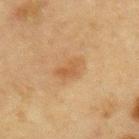<lesion>
<biopsy_status>not biopsied; imaged during a skin examination</biopsy_status>
<patient>
  <sex>male</sex>
  <age_approx>65</age_approx>
</patient>
<lesion_size>
  <long_diameter_mm_approx>3.5</long_diameter_mm_approx>
</lesion_size>
<site>arm</site>
<image>
  <source>total-body photography crop</source>
  <field_of_view_mm>15</field_of_view_mm>
</image>
<lighting>cross-polarized</lighting>
</lesion>